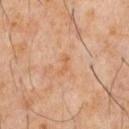Acquisition and patient details:
The patient is a male approximately 60 years of age. Cropped from a whole-body photographic skin survey; the tile spans about 15 mm. Located on the chest.